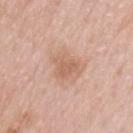Findings:
- notes — no biopsy performed (imaged during a skin exam)
- diameter — ≈4.5 mm
- lighting — white-light illumination
- image — total-body-photography crop, ~15 mm field of view
- subject — male, aged 58 to 62
- anatomic site — the upper back